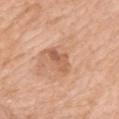Case summary:
* follow-up · imaged on a skin check; not biopsied
* lighting · white-light illumination
* acquisition · total-body-photography crop, ~15 mm field of view
* patient · female, approximately 70 years of age
* size · about 4 mm
* anatomic site · the mid back